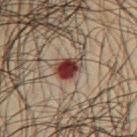  biopsy_status: not biopsied; imaged during a skin examination
  lesion_size:
    long_diameter_mm_approx: 3.0
  patient:
    sex: male
    age_approx: 65
  site: chest
  lighting: cross-polarized
  automated_metrics:
    cielab_L: 30
    cielab_a: 20
    cielab_b: 20
    vs_skin_darker_L: 16.0
    vs_skin_contrast_norm: 15.0
    nevus_likeness_0_100: 0
    lesion_detection_confidence_0_100: 100
  image:
    source: total-body photography crop
    field_of_view_mm: 15